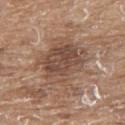  biopsy_status: not biopsied; imaged during a skin examination
  lighting: white-light
  lesion_size:
    long_diameter_mm_approx: 7.0
  site: upper back
  image:
    source: total-body photography crop
    field_of_view_mm: 15
  patient:
    sex: male
    age_approx: 80